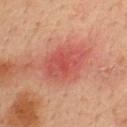Captured during whole-body skin photography for melanoma surveillance; the lesion was not biopsied. The patient is a male in their mid-30s. The lesion is located on the upper back. The total-body-photography lesion software estimated an area of roughly 15 mm². The analysis additionally found an average lesion color of about L≈48 a*≈32 b*≈28 (CIELAB), about 8 CIELAB-L* units darker than the surrounding skin, and a normalized border contrast of about 6. It also reported a classifier nevus-likeness of about 0/100 and a detector confidence of about 100 out of 100 that the crop contains a lesion. Captured under cross-polarized illumination. A lesion tile, about 15 mm wide, cut from a 3D total-body photograph. The recorded lesion diameter is about 5 mm.